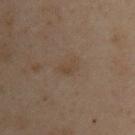patient: male, aged 53–57 | image-analysis metrics: a shape eccentricity near 0.8 and two-axis asymmetry of about 0.25; an automated nevus-likeness rating near 0 out of 100 and a lesion-detection confidence of about 100/100 | anatomic site: the right upper arm | image source: ~15 mm tile from a whole-body skin photo | size: about 3 mm | lighting: cross-polarized illumination.